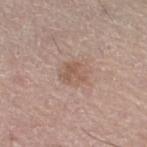Assessment:
The lesion was photographed on a routine skin check and not biopsied; there is no pathology result.
Background:
The lesion is located on the right leg. Imaged with white-light lighting. A male subject, aged 68 to 72. An algorithmic analysis of the crop reported an area of roughly 6.5 mm², an eccentricity of roughly 0.55, and a symmetry-axis asymmetry near 0.15. It also reported a mean CIELAB color near L≈56 a*≈18 b*≈26 and a lesion-to-skin contrast of about 5.5 (normalized; higher = more distinct). And it measured a border-irregularity rating of about 1.5/10, a color-variation rating of about 3.5/10, and peripheral color asymmetry of about 1.5. The analysis additionally found an automated nevus-likeness rating near 0 out of 100 and a detector confidence of about 100 out of 100 that the crop contains a lesion. The recorded lesion diameter is about 3 mm. A 15 mm crop from a total-body photograph taken for skin-cancer surveillance.